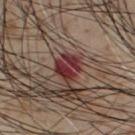biopsy_status: not biopsied; imaged during a skin examination
site: chest
patient:
  sex: male
  age_approx: 55
image:
  source: total-body photography crop
  field_of_view_mm: 15